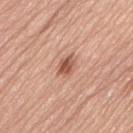follow-up: total-body-photography surveillance lesion; no biopsy
lesion diameter: about 2.5 mm
image: ~15 mm tile from a whole-body skin photo
patient: male, aged 73–77
location: the lower back
illumination: white-light